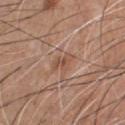Q: Was this lesion biopsied?
A: catalogued during a skin exam; not biopsied
Q: Where on the body is the lesion?
A: the chest
Q: Patient demographics?
A: male, aged 58–62
Q: Lesion size?
A: about 3 mm
Q: How was this image acquired?
A: 15 mm crop, total-body photography